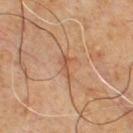Recorded during total-body skin imaging; not selected for excision or biopsy.
A male patient, about 65 years old.
The total-body-photography lesion software estimated a mean CIELAB color near L≈46 a*≈19 b*≈30, a lesion–skin lightness drop of about 7, and a normalized lesion–skin contrast near 5.5. The analysis additionally found a border-irregularity index near 5/10, internal color variation of about 1 on a 0–10 scale, and peripheral color asymmetry of about 0.5. And it measured a lesion-detection confidence of about 100/100.
The tile uses cross-polarized illumination.
The lesion's longest dimension is about 3 mm.
From the chest.
A region of skin cropped from a whole-body photographic capture, roughly 15 mm wide.